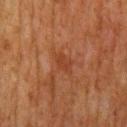* notes — no biopsy performed (imaged during a skin exam)
* lesion diameter — ≈2.5 mm
* TBP lesion metrics — a lesion area of about 3.5 mm², a shape eccentricity near 0.75, and a symmetry-axis asymmetry near 0.2; an average lesion color of about L≈32 a*≈22 b*≈29 (CIELAB), roughly 5 lightness units darker than nearby skin, and a normalized border contrast of about 5.5; border irregularity of about 2 on a 0–10 scale and a peripheral color-asymmetry measure near 0.5; a classifier nevus-likeness of about 0/100 and a lesion-detection confidence of about 100/100
* tile lighting — cross-polarized
* subject — male, aged around 80
* image — 15 mm crop, total-body photography
* body site — the mid back